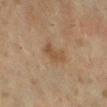Findings:
- biopsy status · total-body-photography surveillance lesion; no biopsy
- patient · female, in their mid- to late 50s
- tile lighting · cross-polarized
- body site · the right lower leg
- image source · total-body-photography crop, ~15 mm field of view